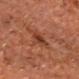Assessment:
This lesion was catalogued during total-body skin photography and was not selected for biopsy.